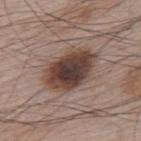Q: Was this lesion biopsied?
A: no biopsy performed (imaged during a skin exam)
Q: What lighting was used for the tile?
A: white-light illumination
Q: Who is the patient?
A: male, approximately 65 years of age
Q: What is the anatomic site?
A: the upper back
Q: Automated lesion metrics?
A: an average lesion color of about L≈41 a*≈17 b*≈21 (CIELAB), roughly 16 lightness units darker than nearby skin, and a normalized border contrast of about 12
Q: What kind of image is this?
A: total-body-photography crop, ~15 mm field of view